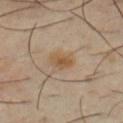{
  "biopsy_status": "not biopsied; imaged during a skin examination",
  "lighting": "cross-polarized",
  "lesion_size": {
    "long_diameter_mm_approx": 3.0
  },
  "patient": {
    "sex": "male",
    "age_approx": 40
  },
  "image": {
    "source": "total-body photography crop",
    "field_of_view_mm": 15
  },
  "site": "chest"
}